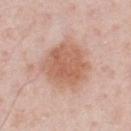Assessment: Part of a total-body skin-imaging series; this lesion was reviewed on a skin check and was not flagged for biopsy. Context: The lesion is on the chest. This image is a 15 mm lesion crop taken from a total-body photograph. A male patient, aged 58 to 62. The lesion's longest dimension is about 5.5 mm. The total-body-photography lesion software estimated a footprint of about 23 mm² and a shape eccentricity near 0.25. The analysis additionally found a lesion–skin lightness drop of about 10 and a normalized border contrast of about 7.5. And it measured border irregularity of about 2 on a 0–10 scale, internal color variation of about 3.5 on a 0–10 scale, and radial color variation of about 1. And it measured a lesion-detection confidence of about 100/100. The tile uses white-light illumination.